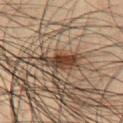follow-up: no biopsy performed (imaged during a skin exam); location: the chest; patient: male, aged approximately 50; imaging modality: ~15 mm crop, total-body skin-cancer survey; lesion size: ≈5 mm.